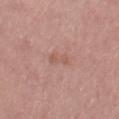Q: Was a biopsy performed?
A: imaged on a skin check; not biopsied
Q: Illumination type?
A: white-light illumination
Q: How was this image acquired?
A: 15 mm crop, total-body photography
Q: Who is the patient?
A: female, aged 63 to 67
Q: Lesion size?
A: about 2.5 mm
Q: Lesion location?
A: the right thigh